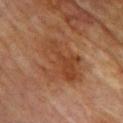notes = catalogued during a skin exam; not biopsied | image source = ~15 mm tile from a whole-body skin photo | patient = female, aged around 80 | location = the upper back.